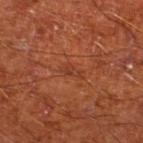workup: no biopsy performed (imaged during a skin exam)
acquisition: ~15 mm crop, total-body skin-cancer survey
location: the left lower leg
lesion diameter: about 2.5 mm
patient: male, in their mid- to late 60s
lighting: cross-polarized